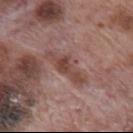Acquisition and patient details: Cropped from a whole-body photographic skin survey; the tile spans about 15 mm. A male subject, approximately 70 years of age. The lesion is located on the mid back.